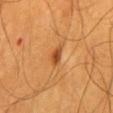Q: Was a biopsy performed?
A: no biopsy performed (imaged during a skin exam)
Q: How was this image acquired?
A: ~15 mm crop, total-body skin-cancer survey
Q: Patient demographics?
A: male, aged 58 to 62
Q: Automated lesion metrics?
A: an area of roughly 3 mm² and a shape-asymmetry score of about 0.3 (0 = symmetric); about 12 CIELAB-L* units darker than the surrounding skin and a lesion-to-skin contrast of about 8.5 (normalized; higher = more distinct); a classifier nevus-likeness of about 90/100 and a detector confidence of about 100 out of 100 that the crop contains a lesion
Q: What is the anatomic site?
A: the back
Q: Lesion size?
A: about 2 mm